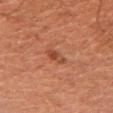<tbp_lesion>
  <biopsy_status>not biopsied; imaged during a skin examination</biopsy_status>
  <patient>
    <sex>male</sex>
    <age_approx>50</age_approx>
  </patient>
  <site>right upper arm</site>
  <image>
    <source>total-body photography crop</source>
    <field_of_view_mm>15</field_of_view_mm>
  </image>
  <lighting>cross-polarized</lighting>
</tbp_lesion>